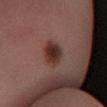Impression: Imaged during a routine full-body skin examination; the lesion was not biopsied and no histopathology is available. Acquisition and patient details: The tile uses cross-polarized illumination. A male subject aged 53–57. The lesion is on the right lower leg. Cropped from a total-body skin-imaging series; the visible field is about 15 mm. Measured at roughly 3 mm in maximum diameter.